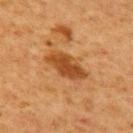{
  "biopsy_status": "not biopsied; imaged during a skin examination",
  "patient": {
    "sex": "female",
    "age_approx": 50
  },
  "image": {
    "source": "total-body photography crop",
    "field_of_view_mm": 15
  },
  "site": "upper back",
  "lesion_size": {
    "long_diameter_mm_approx": 5.0
  },
  "automated_metrics": {
    "area_mm2_approx": 10.0,
    "eccentricity": 0.9,
    "shape_asymmetry": 0.2,
    "border_irregularity_0_10": 2.5,
    "color_variation_0_10": 3.0,
    "nevus_likeness_0_100": 55,
    "lesion_detection_confidence_0_100": 100
  }
}